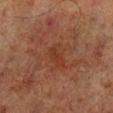Captured during whole-body skin photography for melanoma surveillance; the lesion was not biopsied.
A 15 mm crop from a total-body photograph taken for skin-cancer surveillance.
The patient is a male aged approximately 70.
On the right lower leg.
Approximately 4 mm at its widest.